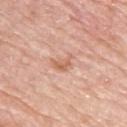A 15 mm close-up extracted from a 3D total-body photography capture. On the left upper arm. A female patient approximately 65 years of age. Measured at roughly 3 mm in maximum diameter. Captured under white-light illumination. The lesion-visualizer software estimated a lesion area of about 4 mm² and a shape eccentricity near 0.75. The analysis additionally found a color-variation rating of about 2.5/10 and a peripheral color-asymmetry measure near 1. The software also gave a lesion-detection confidence of about 100/100.